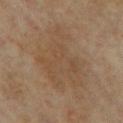workup = catalogued during a skin exam; not biopsied | anatomic site = the chest | imaging modality = ~15 mm tile from a whole-body skin photo | patient = female, aged 58–62 | lesion size = about 10 mm.